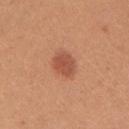Q: Was this lesion biopsied?
A: no biopsy performed (imaged during a skin exam)
Q: Where on the body is the lesion?
A: the right upper arm
Q: What are the patient's age and sex?
A: female, aged 23 to 27
Q: What kind of image is this?
A: 15 mm crop, total-body photography